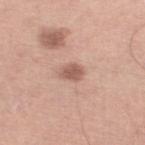  biopsy_status: not biopsied; imaged during a skin examination
  image:
    source: total-body photography crop
    field_of_view_mm: 15
  lighting: white-light
  lesion_size:
    long_diameter_mm_approx: 2.5
  patient:
    sex: male
    age_approx: 50
  site: left lower leg